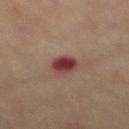– follow-up: catalogued during a skin exam; not biopsied